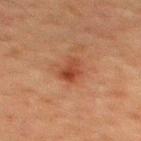Recorded during total-body skin imaging; not selected for excision or biopsy. The lesion is on the back. A male subject, approximately 60 years of age. Cropped from a whole-body photographic skin survey; the tile spans about 15 mm. Automated image analysis of the tile measured a nevus-likeness score of about 55/100 and a lesion-detection confidence of about 100/100. About 3.5 mm across.